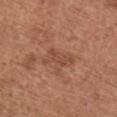workup: catalogued during a skin exam; not biopsied
image: 15 mm crop, total-body photography
lesion size: ≈4 mm
subject: female, aged approximately 50
location: the chest
tile lighting: white-light